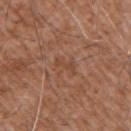Notes:
- biopsy status — no biopsy performed (imaged during a skin exam)
- location — the right upper arm
- acquisition — 15 mm crop, total-body photography
- subject — male, aged approximately 75
- lesion diameter — ≈3 mm